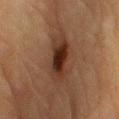Imaged during a routine full-body skin examination; the lesion was not biopsied and no histopathology is available. The lesion is on the arm. A male patient, roughly 85 years of age or older. Cropped from a whole-body photographic skin survey; the tile spans about 15 mm.